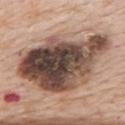Q: Is there a histopathology result?
A: total-body-photography surveillance lesion; no biopsy
Q: How large is the lesion?
A: about 11 mm
Q: Patient demographics?
A: female, roughly 70 years of age
Q: Automated lesion metrics?
A: a color-variation rating of about 10/10 and radial color variation of about 4; a nevus-likeness score of about 5/100 and a detector confidence of about 75 out of 100 that the crop contains a lesion
Q: What lighting was used for the tile?
A: white-light
Q: What is the imaging modality?
A: ~15 mm crop, total-body skin-cancer survey
Q: What is the anatomic site?
A: the upper back